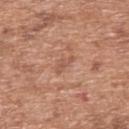| key | value |
|---|---|
| follow-up | catalogued during a skin exam; not biopsied |
| lesion size | ~3 mm (longest diameter) |
| lighting | white-light |
| anatomic site | the upper back |
| image source | 15 mm crop, total-body photography |
| subject | male, aged 63–67 |